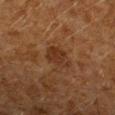notes: no biopsy performed (imaged during a skin exam)
lighting: cross-polarized
lesion size: about 3 mm
patient: male, aged around 60
image source: 15 mm crop, total-body photography
TBP lesion metrics: a nevus-likeness score of about 0/100 and lesion-presence confidence of about 100/100
anatomic site: the arm